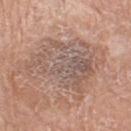Part of a total-body skin-imaging series; this lesion was reviewed on a skin check and was not flagged for biopsy.
About 7.5 mm across.
This is a white-light tile.
The lesion is on the right thigh.
The patient is a female in their mid-70s.
A 15 mm crop from a total-body photograph taken for skin-cancer surveillance.